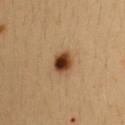This lesion was catalogued during total-body skin photography and was not selected for biopsy. A female subject, roughly 35 years of age. The lesion is located on the upper back. Captured under cross-polarized illumination. This image is a 15 mm lesion crop taken from a total-body photograph. Measured at roughly 2.5 mm in maximum diameter.